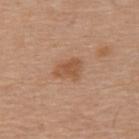Q: Was this lesion biopsied?
A: total-body-photography surveillance lesion; no biopsy
Q: How large is the lesion?
A: ~3.5 mm (longest diameter)
Q: How was the tile lit?
A: white-light
Q: Who is the patient?
A: male, about 65 years old
Q: What did automated image analysis measure?
A: a mean CIELAB color near L≈53 a*≈21 b*≈33, a lesion–skin lightness drop of about 9, and a normalized border contrast of about 6.5; a border-irregularity rating of about 2.5/10 and a color-variation rating of about 2/10
Q: How was this image acquired?
A: ~15 mm crop, total-body skin-cancer survey
Q: What is the anatomic site?
A: the upper back